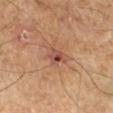Q: Is there a histopathology result?
A: catalogued during a skin exam; not biopsied
Q: What are the patient's age and sex?
A: male, aged 53 to 57
Q: What did automated image analysis measure?
A: a footprint of about 4 mm², an eccentricity of roughly 0.75, and a shape-asymmetry score of about 0.2 (0 = symmetric); a lesion-to-skin contrast of about 7.5 (normalized; higher = more distinct); an automated nevus-likeness rating near 0 out of 100 and a detector confidence of about 100 out of 100 that the crop contains a lesion
Q: How large is the lesion?
A: ~3 mm (longest diameter)
Q: Where on the body is the lesion?
A: the right lower leg
Q: How was this image acquired?
A: total-body-photography crop, ~15 mm field of view